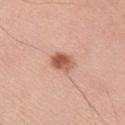  biopsy_status: not biopsied; imaged during a skin examination
  image:
    source: total-body photography crop
    field_of_view_mm: 15
  patient:
    sex: male
    age_approx: 70
  lighting: white-light
  lesion_size:
    long_diameter_mm_approx: 3.0
  site: right upper arm